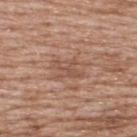Q: Lesion location?
A: the upper back
Q: How was this image acquired?
A: total-body-photography crop, ~15 mm field of view
Q: Automated lesion metrics?
A: internal color variation of about 2 on a 0–10 scale
Q: Illumination type?
A: white-light illumination
Q: Who is the patient?
A: male, aged 48–52
Q: How large is the lesion?
A: ~3.5 mm (longest diameter)